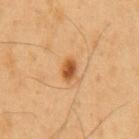Acquisition and patient details:
This is a cross-polarized tile. A 15 mm close-up extracted from a 3D total-body photography capture. Longest diameter approximately 2.5 mm. The lesion is on the chest. A male patient aged 53 to 57.